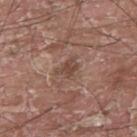Assessment:
The lesion was tiled from a total-body skin photograph and was not biopsied.
Image and clinical context:
Longest diameter approximately 3 mm. From the upper back. Imaged with white-light lighting. A close-up tile cropped from a whole-body skin photograph, about 15 mm across. A male subject approximately 40 years of age. Automated image analysis of the tile measured an average lesion color of about L≈45 a*≈18 b*≈25 (CIELAB) and a lesion–skin lightness drop of about 8. The software also gave a within-lesion color-variation index near 2/10 and peripheral color asymmetry of about 0.5.